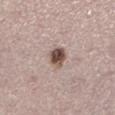{
  "biopsy_status": "not biopsied; imaged during a skin examination",
  "image": {
    "source": "total-body photography crop",
    "field_of_view_mm": 15
  },
  "site": "left lower leg",
  "patient": {
    "sex": "male",
    "age_approx": 60
  }
}